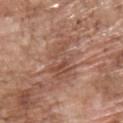Clinical impression: No biopsy was performed on this lesion — it was imaged during a full skin examination and was not determined to be concerning. Image and clinical context: A male patient, aged around 70. Captured under white-light illumination. The lesion-visualizer software estimated a lesion area of about 12 mm², an outline eccentricity of about 0.8 (0 = round, 1 = elongated), and a symmetry-axis asymmetry near 0.3. The software also gave a classifier nevus-likeness of about 0/100 and a detector confidence of about 90 out of 100 that the crop contains a lesion. Cropped from a total-body skin-imaging series; the visible field is about 15 mm. On the back. Approximately 5.5 mm at its widest.